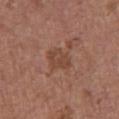{
  "biopsy_status": "not biopsied; imaged during a skin examination",
  "lighting": "white-light",
  "automated_metrics": {
    "eccentricity": 0.6,
    "shape_asymmetry": 0.2,
    "cielab_L": 44,
    "cielab_a": 21,
    "cielab_b": 28,
    "vs_skin_darker_L": 7.0,
    "vs_skin_contrast_norm": 6.0
  },
  "patient": {
    "sex": "female",
    "age_approx": 65
  },
  "image": {
    "source": "total-body photography crop",
    "field_of_view_mm": 15
  },
  "site": "chest",
  "lesion_size": {
    "long_diameter_mm_approx": 3.0
  }
}